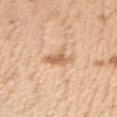Captured during whole-body skin photography for melanoma surveillance; the lesion was not biopsied.
The subject is a female aged around 40.
A 15 mm crop from a total-body photograph taken for skin-cancer surveillance.
The lesion is on the right upper arm.
Imaged with white-light lighting.